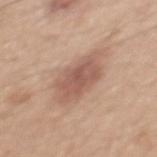| feature | finding |
|---|---|
| biopsy status | no biopsy performed (imaged during a skin exam) |
| body site | the mid back |
| imaging modality | ~15 mm crop, total-body skin-cancer survey |
| lesion size | ~6.5 mm (longest diameter) |
| patient | male, roughly 45 years of age |
| illumination | white-light illumination |